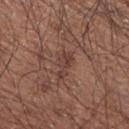The lesion was photographed on a routine skin check and not biopsied; there is no pathology result.
From the right upper arm.
Cropped from a whole-body photographic skin survey; the tile spans about 15 mm.
A male subject aged around 65.